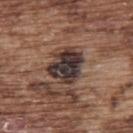Q: Was this lesion biopsied?
A: imaged on a skin check; not biopsied
Q: How was this image acquired?
A: ~15 mm crop, total-body skin-cancer survey
Q: Where on the body is the lesion?
A: the upper back
Q: Who is the patient?
A: male, aged approximately 75